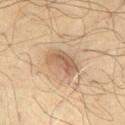Image and clinical context: The lesion is located on the chest. Cropped from a total-body skin-imaging series; the visible field is about 15 mm. The lesion's longest dimension is about 4.5 mm. A male subject in their 50s.